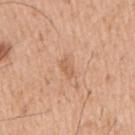Recorded during total-body skin imaging; not selected for excision or biopsy.
Longest diameter approximately 2.5 mm.
The lesion is located on the right upper arm.
This is a white-light tile.
A close-up tile cropped from a whole-body skin photograph, about 15 mm across.
A male subject, aged 38–42.